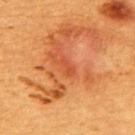biopsy status: total-body-photography surveillance lesion; no biopsy | subject: female, aged 53–57 | size: ~2 mm (longest diameter) | body site: the back | image source: total-body-photography crop, ~15 mm field of view | tile lighting: cross-polarized illumination.